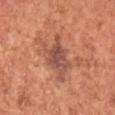body site=the chest
image-analysis metrics=a border-irregularity rating of about 6/10, internal color variation of about 4 on a 0–10 scale, and a peripheral color-asymmetry measure near 1
acquisition=~15 mm tile from a whole-body skin photo
subject=male, aged approximately 65
lesion diameter=≈5 mm
illumination=white-light illumination